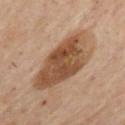Part of a total-body skin-imaging series; this lesion was reviewed on a skin check and was not flagged for biopsy.
A male subject, in their 50s.
On the chest.
A lesion tile, about 15 mm wide, cut from a 3D total-body photograph.